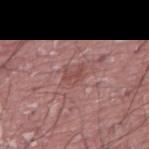acquisition = ~15 mm tile from a whole-body skin photo
patient = male, roughly 40 years of age
image-analysis metrics = a mean CIELAB color near L≈47 a*≈24 b*≈22 and roughly 7 lightness units darker than nearby skin; border irregularity of about 6 on a 0–10 scale and a color-variation rating of about 0/10; a detector confidence of about 85 out of 100 that the crop contains a lesion
lesion diameter = about 2.5 mm
illumination = white-light illumination
anatomic site = the right thigh